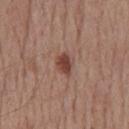{"patient": {"sex": "female", "age_approx": 55}, "lighting": "white-light", "image": {"source": "total-body photography crop", "field_of_view_mm": 15}, "site": "head or neck", "lesion_size": {"long_diameter_mm_approx": 3.0}}